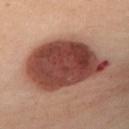Q: Patient demographics?
A: female, in their mid- to late 40s
Q: Lesion location?
A: the front of the torso
Q: Lesion size?
A: ~9.5 mm (longest diameter)
Q: Automated lesion metrics?
A: a lesion area of about 41 mm², an outline eccentricity of about 0.8 (0 = round, 1 = elongated), and two-axis asymmetry of about 0.2; a border-irregularity index near 2/10, a color-variation rating of about 5.5/10, and peripheral color asymmetry of about 2; a lesion-detection confidence of about 100/100
Q: What is the imaging modality?
A: 15 mm crop, total-body photography
Q: What lighting was used for the tile?
A: cross-polarized